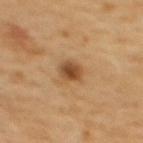Impression:
Imaged during a routine full-body skin examination; the lesion was not biopsied and no histopathology is available.
Acquisition and patient details:
Captured under cross-polarized illumination. A female subject, in their mid- to late 60s. Located on the upper back. Approximately 2.5 mm at its widest. A roughly 15 mm field-of-view crop from a total-body skin photograph.